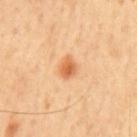Recorded during total-body skin imaging; not selected for excision or biopsy. The tile uses cross-polarized illumination. The patient is a male about 55 years old. An algorithmic analysis of the crop reported an area of roughly 4 mm², an eccentricity of roughly 0.6, and a shape-asymmetry score of about 0.2 (0 = symmetric). The software also gave a mean CIELAB color near L≈64 a*≈27 b*≈43, about 12 CIELAB-L* units darker than the surrounding skin, and a normalized border contrast of about 8. The analysis additionally found a classifier nevus-likeness of about 95/100 and lesion-presence confidence of about 100/100. From the mid back. The lesion's longest dimension is about 2.5 mm. A region of skin cropped from a whole-body photographic capture, roughly 15 mm wide.